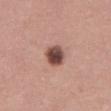Recorded during total-body skin imaging; not selected for excision or biopsy. The tile uses white-light illumination. The patient is a male aged approximately 55. A roughly 15 mm field-of-view crop from a total-body skin photograph. Approximately 3 mm at its widest. Located on the abdomen. The total-body-photography lesion software estimated a lesion color around L≈46 a*≈21 b*≈23 in CIELAB, roughly 18 lightness units darker than nearby skin, and a normalized border contrast of about 12.5.